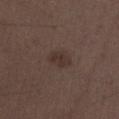No biopsy was performed on this lesion — it was imaged during a full skin examination and was not determined to be concerning. A male patient, aged around 50. Measured at roughly 3 mm in maximum diameter. This is a white-light tile. The lesion is located on the left lower leg. Cropped from a total-body skin-imaging series; the visible field is about 15 mm.